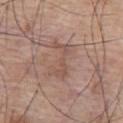Q: Is there a histopathology result?
A: no biopsy performed (imaged during a skin exam)
Q: What kind of image is this?
A: total-body-photography crop, ~15 mm field of view
Q: Lesion location?
A: the chest
Q: What are the patient's age and sex?
A: male, approximately 75 years of age
Q: How was the tile lit?
A: white-light